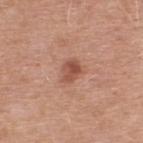Captured during whole-body skin photography for melanoma surveillance; the lesion was not biopsied. From the upper back. An algorithmic analysis of the crop reported border irregularity of about 2 on a 0–10 scale, a color-variation rating of about 4.5/10, and radial color variation of about 1.5. The software also gave a classifier nevus-likeness of about 75/100 and lesion-presence confidence of about 100/100. The lesion's longest dimension is about 3 mm. A male patient roughly 50 years of age. Captured under white-light illumination. A 15 mm close-up extracted from a 3D total-body photography capture.